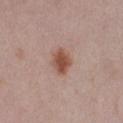{"biopsy_status": "not biopsied; imaged during a skin examination"}